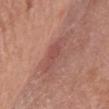A 15 mm crop from a total-body photograph taken for skin-cancer surveillance. The lesion is on the chest. The recorded lesion diameter is about 5 mm. A female subject about 60 years old. Captured under white-light illumination.